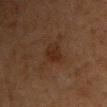This lesion was catalogued during total-body skin photography and was not selected for biopsy. Located on the chest. Imaged with cross-polarized lighting. Approximately 2.5 mm at its widest. The subject is a female roughly 60 years of age. A 15 mm crop from a total-body photograph taken for skin-cancer surveillance.